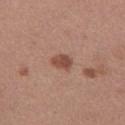This lesion was catalogued during total-body skin photography and was not selected for biopsy. Located on the right thigh. This is a white-light tile. The patient is a female aged 33–37. An algorithmic analysis of the crop reported a mean CIELAB color near L≈48 a*≈23 b*≈27, roughly 11 lightness units darker than nearby skin, and a lesion-to-skin contrast of about 8.5 (normalized; higher = more distinct). And it measured a border-irregularity rating of about 1.5/10, internal color variation of about 2 on a 0–10 scale, and a peripheral color-asymmetry measure near 0.5. Measured at roughly 2.5 mm in maximum diameter. A 15 mm crop from a total-body photograph taken for skin-cancer surveillance.